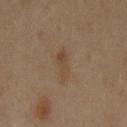Assessment:
The lesion was photographed on a routine skin check and not biopsied; there is no pathology result.
Acquisition and patient details:
Located on the front of the torso. An algorithmic analysis of the crop reported a lesion area of about 5 mm², an eccentricity of roughly 0.95, and two-axis asymmetry of about 0.3. And it measured a nevus-likeness score of about 10/100 and lesion-presence confidence of about 100/100. The lesion's longest dimension is about 4 mm. The patient is a male roughly 60 years of age. This image is a 15 mm lesion crop taken from a total-body photograph.